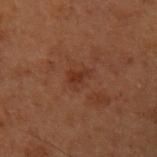{
  "biopsy_status": "not biopsied; imaged during a skin examination",
  "site": "left upper arm",
  "image": {
    "source": "total-body photography crop",
    "field_of_view_mm": 15
  },
  "patient": {
    "sex": "male",
    "age_approx": 70
  }
}